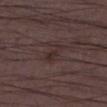follow-up: imaged on a skin check; not biopsied | body site: the right lower leg | image source: ~15 mm tile from a whole-body skin photo | subject: male, roughly 50 years of age | diameter: ~3 mm (longest diameter).